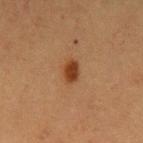<record>
<biopsy_status>not biopsied; imaged during a skin examination</biopsy_status>
<lighting>cross-polarized</lighting>
<site>arm</site>
<lesion_size>
  <long_diameter_mm_approx>2.5</long_diameter_mm_approx>
</lesion_size>
<image>
  <source>total-body photography crop</source>
  <field_of_view_mm>15</field_of_view_mm>
</image>
<patient>
  <sex>male</sex>
  <age_approx>40</age_approx>
</patient>
<automated_metrics>
  <cielab_L>32</cielab_L>
  <cielab_a>22</cielab_a>
  <cielab_b>31</cielab_b>
  <vs_skin_contrast_norm>10.5</vs_skin_contrast_norm>
  <nevus_likeness_0_100>100</nevus_likeness_0_100>
  <lesion_detection_confidence_0_100>100</lesion_detection_confidence_0_100>
</automated_metrics>
</record>